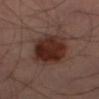Notes:
* follow-up: catalogued during a skin exam; not biopsied
* lesion diameter: about 6 mm
* image source: ~15 mm crop, total-body skin-cancer survey
* site: the right thigh